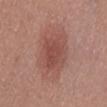patient: female, aged around 50
acquisition: total-body-photography crop, ~15 mm field of view
body site: the front of the torso
size: ~4 mm (longest diameter)